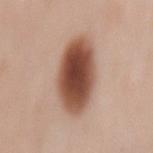biopsy_status: not biopsied; imaged during a skin examination
site: mid back
image:
  source: total-body photography crop
  field_of_view_mm: 15
patient:
  sex: female
  age_approx: 50
automated_metrics:
  eccentricity: 0.8
  shape_asymmetry: 0.1
  nevus_likeness_0_100: 100
  lesion_detection_confidence_0_100: 100
lesion_size:
  long_diameter_mm_approx: 7.0
lighting: white-light